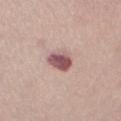Impression:
This lesion was catalogued during total-body skin photography and was not selected for biopsy.
Acquisition and patient details:
Measured at roughly 3.5 mm in maximum diameter. Imaged with white-light lighting. A female patient aged approximately 35. The lesion is on the chest. A 15 mm close-up extracted from a 3D total-body photography capture.